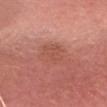No biopsy was performed on this lesion — it was imaged during a full skin examination and was not determined to be concerning.
A female subject aged around 40.
A roughly 15 mm field-of-view crop from a total-body skin photograph.
On the head or neck.